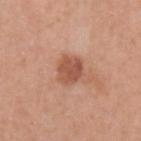| field | value |
|---|---|
| notes | catalogued during a skin exam; not biopsied |
| acquisition | total-body-photography crop, ~15 mm field of view |
| location | the left upper arm |
| patient | female, about 50 years old |